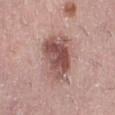notes: total-body-photography surveillance lesion; no biopsy | acquisition: 15 mm crop, total-body photography | location: the right lower leg | subject: female, aged 38 to 42.